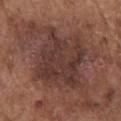The lesion is on the chest. A 15 mm close-up extracted from a 3D total-body photography capture. Approximately 8 mm at its widest. This is a white-light tile. Automated tile analysis of the lesion measured a lesion area of about 37 mm², a shape eccentricity near 0.55, and a shape-asymmetry score of about 0.25 (0 = symmetric). It also reported border irregularity of about 4 on a 0–10 scale and radial color variation of about 1.5. The subject is a male about 75 years old.